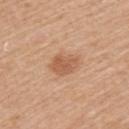Assessment:
Part of a total-body skin-imaging series; this lesion was reviewed on a skin check and was not flagged for biopsy.
Background:
A female patient, roughly 50 years of age. This is a white-light tile. A roughly 15 mm field-of-view crop from a total-body skin photograph. Located on the arm. Longest diameter approximately 3.5 mm.